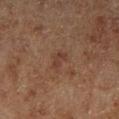Q: How was this image acquired?
A: total-body-photography crop, ~15 mm field of view
Q: Patient demographics?
A: male, in their mid-50s
Q: Where on the body is the lesion?
A: the leg
Q: How large is the lesion?
A: ~2.5 mm (longest diameter)